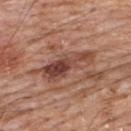The lesion was tiled from a total-body skin photograph and was not biopsied.
A 15 mm close-up extracted from a 3D total-body photography capture.
A male subject approximately 80 years of age.
The tile uses white-light illumination.
On the upper back.